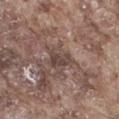Assessment:
Captured during whole-body skin photography for melanoma surveillance; the lesion was not biopsied.
Image and clinical context:
The lesion is located on the right thigh. Automated tile analysis of the lesion measured a normalized border contrast of about 7. The analysis additionally found border irregularity of about 3 on a 0–10 scale, internal color variation of about 2.5 on a 0–10 scale, and a peripheral color-asymmetry measure near 1. It also reported a classifier nevus-likeness of about 0/100 and a detector confidence of about 65 out of 100 that the crop contains a lesion. Longest diameter approximately 3 mm. The tile uses white-light illumination. Cropped from a whole-body photographic skin survey; the tile spans about 15 mm. A male subject, aged around 75.